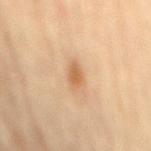Part of a total-body skin-imaging series; this lesion was reviewed on a skin check and was not flagged for biopsy. The subject is a female approximately 80 years of age. Captured under cross-polarized illumination. Approximately 3 mm at its widest. A region of skin cropped from a whole-body photographic capture, roughly 15 mm wide. Located on the mid back.